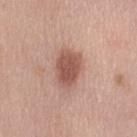workup: no biopsy performed (imaged during a skin exam); image source: ~15 mm crop, total-body skin-cancer survey; anatomic site: the left thigh; subject: female, in their 40s.